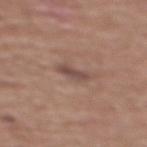The lesion was photographed on a routine skin check and not biopsied; there is no pathology result.
Located on the back.
The patient is a male in their mid- to late 70s.
Automated tile analysis of the lesion measured an average lesion color of about L≈47 a*≈18 b*≈22 (CIELAB), roughly 9 lightness units darker than nearby skin, and a normalized border contrast of about 7.
This image is a 15 mm lesion crop taken from a total-body photograph.
Imaged with white-light lighting.
Approximately 3 mm at its widest.